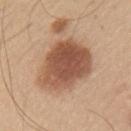Recorded during total-body skin imaging; not selected for excision or biopsy. Located on the arm. A male subject aged 43–47. A 15 mm close-up extracted from a 3D total-body photography capture.